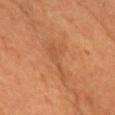<lesion>
  <biopsy_status>not biopsied; imaged during a skin examination</biopsy_status>
  <image>
    <source>total-body photography crop</source>
    <field_of_view_mm>15</field_of_view_mm>
  </image>
  <site>chest</site>
  <automated_metrics>
    <vs_skin_darker_L>5.0</vs_skin_darker_L>
    <vs_skin_contrast_norm>4.5</vs_skin_contrast_norm>
    <nevus_likeness_0_100>0</nevus_likeness_0_100>
    <lesion_detection_confidence_0_100>85</lesion_detection_confidence_0_100>
  </automated_metrics>
  <patient>
    <sex>female</sex>
    <age_approx>55</age_approx>
  </patient>
  <lighting>cross-polarized</lighting>
  <lesion_size>
    <long_diameter_mm_approx>6.0</long_diameter_mm_approx>
  </lesion_size>
</lesion>